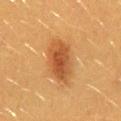| feature | finding |
|---|---|
| follow-up | catalogued during a skin exam; not biopsied |